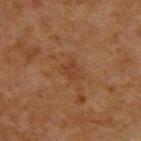follow-up: no biopsy performed (imaged during a skin exam)
lesion diameter: about 2.5 mm
image-analysis metrics: a lesion-detection confidence of about 100/100
subject: male, about 50 years old
anatomic site: the upper back
lighting: cross-polarized
image source: 15 mm crop, total-body photography